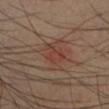Imaged during a routine full-body skin examination; the lesion was not biopsied and no histopathology is available. A 15 mm crop from a total-body photograph taken for skin-cancer surveillance. The total-body-photography lesion software estimated an area of roughly 5 mm², an outline eccentricity of about 0.6 (0 = round, 1 = elongated), and two-axis asymmetry of about 0.4. The software also gave a lesion color around L≈38 a*≈22 b*≈25 in CIELAB, about 5 CIELAB-L* units darker than the surrounding skin, and a normalized border contrast of about 5. A male subject in their 40s. Captured under cross-polarized illumination. The lesion's longest dimension is about 3 mm. The lesion is on the left lower leg.